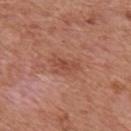Captured during whole-body skin photography for melanoma surveillance; the lesion was not biopsied.